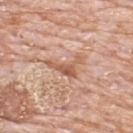Context: From the upper back. This is a white-light tile. About 4.5 mm across. Cropped from a whole-body photographic skin survey; the tile spans about 15 mm. A male subject, about 80 years old.